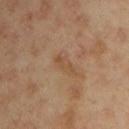Assessment:
This lesion was catalogued during total-body skin photography and was not selected for biopsy.
Image and clinical context:
On the left upper arm. A close-up tile cropped from a whole-body skin photograph, about 15 mm across. About 3 mm across. The subject is a male aged around 45. The tile uses cross-polarized illumination.